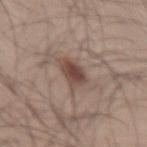- workup · total-body-photography surveillance lesion; no biopsy
- tile lighting · white-light illumination
- lesion size · about 4 mm
- anatomic site · the back
- automated lesion analysis · an outline eccentricity of about 0.75 (0 = round, 1 = elongated) and a symmetry-axis asymmetry near 0.3; a lesion color around L≈46 a*≈16 b*≈23 in CIELAB, roughly 11 lightness units darker than nearby skin, and a lesion-to-skin contrast of about 8.5 (normalized; higher = more distinct)
- image · ~15 mm tile from a whole-body skin photo
- patient · male, aged 48 to 52